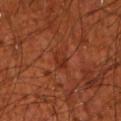workup: total-body-photography surveillance lesion; no biopsy | diameter: about 2.5 mm | patient: male, aged 68–72 | site: the left upper arm | TBP lesion metrics: an outline eccentricity of about 0.75 (0 = round, 1 = elongated) and a shape-asymmetry score of about 0.2 (0 = symmetric); roughly 6 lightness units darker than nearby skin and a normalized lesion–skin contrast near 6; a border-irregularity index near 2/10, internal color variation of about 3.5 on a 0–10 scale, and radial color variation of about 1.5 | acquisition: 15 mm crop, total-body photography.